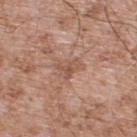Clinical impression: This lesion was catalogued during total-body skin photography and was not selected for biopsy. Background: A close-up tile cropped from a whole-body skin photograph, about 15 mm across. On the upper back. Imaged with white-light lighting. About 3 mm across. A male subject, aged 53 to 57.